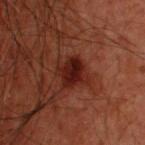workup = imaged on a skin check; not biopsied.